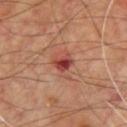On the chest. The subject is a male aged approximately 60. A lesion tile, about 15 mm wide, cut from a 3D total-body photograph.